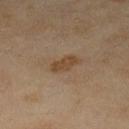notes: no biopsy performed (imaged during a skin exam) | image: ~15 mm crop, total-body skin-cancer survey | image-analysis metrics: a footprint of about 5 mm² and an eccentricity of roughly 0.9; a border-irregularity index near 3.5/10 and a peripheral color-asymmetry measure near 0.5 | patient: female, in their 60s | site: the left lower leg.